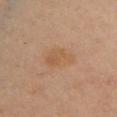Notes:
• notes — imaged on a skin check; not biopsied
• location — the front of the torso
• subject — female, roughly 40 years of age
• tile lighting — cross-polarized illumination
• image — ~15 mm crop, total-body skin-cancer survey
• image-analysis metrics — a lesion area of about 8 mm² and a shape-asymmetry score of about 0.25 (0 = symmetric); a lesion color around L≈56 a*≈19 b*≈35 in CIELAB; border irregularity of about 2.5 on a 0–10 scale, a within-lesion color-variation index near 3/10, and radial color variation of about 1; a nevus-likeness score of about 25/100 and lesion-presence confidence of about 100/100